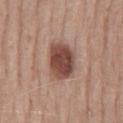<lesion>
  <biopsy_status>not biopsied; imaged during a skin examination</biopsy_status>
  <image>
    <source>total-body photography crop</source>
    <field_of_view_mm>15</field_of_view_mm>
  </image>
  <automated_metrics>
    <area_mm2_approx>13.0</area_mm2_approx>
    <eccentricity>0.65</eccentricity>
    <shape_asymmetry>0.15</shape_asymmetry>
    <cielab_L>46</cielab_L>
    <cielab_a>20</cielab_a>
    <cielab_b>25</cielab_b>
    <vs_skin_darker_L>15.0</vs_skin_darker_L>
    <vs_skin_contrast_norm>11.0</vs_skin_contrast_norm>
    <lesion_detection_confidence_0_100>100</lesion_detection_confidence_0_100>
  </automated_metrics>
  <site>mid back</site>
  <patient>
    <sex>male</sex>
    <age_approx>60</age_approx>
  </patient>
  <lighting>white-light</lighting>
</lesion>